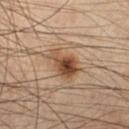Findings:
• workup: total-body-photography surveillance lesion; no biopsy
• lesion diameter: ≈4 mm
• patient: male, in their mid-50s
• automated lesion analysis: a footprint of about 9 mm² and a symmetry-axis asymmetry near 0.35; a lesion color around L≈38 a*≈16 b*≈27 in CIELAB, about 11 CIELAB-L* units darker than the surrounding skin, and a lesion-to-skin contrast of about 9.5 (normalized; higher = more distinct); a classifier nevus-likeness of about 95/100 and a lesion-detection confidence of about 100/100
• image source: ~15 mm tile from a whole-body skin photo
• location: the leg
• lighting: cross-polarized